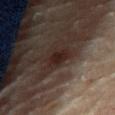follow-up=imaged on a skin check; not biopsied | lighting=cross-polarized | lesion size=about 3.5 mm | anatomic site=the right thigh | patient=male, about 85 years old or older | image=total-body-photography crop, ~15 mm field of view.